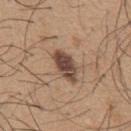Impression: The lesion was photographed on a routine skin check and not biopsied; there is no pathology result. Background: A male patient, about 65 years old. The recorded lesion diameter is about 4.5 mm. From the chest. A 15 mm close-up tile from a total-body photography series done for melanoma screening.